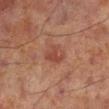Captured during whole-body skin photography for melanoma surveillance; the lesion was not biopsied. On the left lower leg. Approximately 3 mm at its widest. The patient is a male aged 68 to 72. This is a cross-polarized tile. A 15 mm close-up tile from a total-body photography series done for melanoma screening.